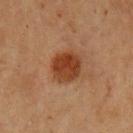biopsy_status: not biopsied; imaged during a skin examination
patient:
  sex: female
  age_approx: 70
lesion_size:
  long_diameter_mm_approx: 3.5
lighting: cross-polarized
automated_metrics:
  area_mm2_approx: 11.0
  eccentricity: 0.3
  shape_asymmetry: 0.1
  border_irregularity_0_10: 1.0
  color_variation_0_10: 4.0
  peripheral_color_asymmetry: 1.5
  nevus_likeness_0_100: 100
  lesion_detection_confidence_0_100: 100
site: left upper arm
image:
  source: total-body photography crop
  field_of_view_mm: 15